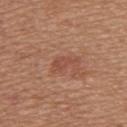Q: Is there a histopathology result?
A: catalogued during a skin exam; not biopsied
Q: What is the anatomic site?
A: the upper back
Q: Automated lesion metrics?
A: a lesion area of about 3.5 mm² and a shape-asymmetry score of about 0.45 (0 = symmetric); roughly 7 lightness units darker than nearby skin and a normalized lesion–skin contrast near 5.5; a lesion-detection confidence of about 100/100
Q: How large is the lesion?
A: about 3.5 mm
Q: Who is the patient?
A: female, aged 53 to 57
Q: How was this image acquired?
A: total-body-photography crop, ~15 mm field of view